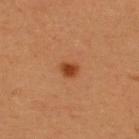<lesion>
<biopsy_status>not biopsied; imaged during a skin examination</biopsy_status>
<site>upper back</site>
<lesion_size>
  <long_diameter_mm_approx>2.0</long_diameter_mm_approx>
</lesion_size>
<image>
  <source>total-body photography crop</source>
  <field_of_view_mm>15</field_of_view_mm>
</image>
<patient>
  <sex>male</sex>
  <age_approx>35</age_approx>
</patient>
</lesion>